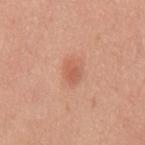Impression:
Imaged during a routine full-body skin examination; the lesion was not biopsied and no histopathology is available.
Context:
Captured under white-light illumination. A male subject aged around 50. The recorded lesion diameter is about 2.5 mm. The total-body-photography lesion software estimated a classifier nevus-likeness of about 90/100. A 15 mm close-up extracted from a 3D total-body photography capture. The lesion is located on the mid back.